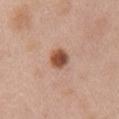This lesion was catalogued during total-body skin photography and was not selected for biopsy. Captured under white-light illumination. A 15 mm close-up extracted from a 3D total-body photography capture. From the right upper arm. The patient is a female aged 48–52. About 2.5 mm across.